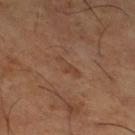notes: total-body-photography surveillance lesion; no biopsy